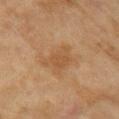– biopsy status: total-body-photography surveillance lesion; no biopsy
– TBP lesion metrics: a lesion area of about 9 mm² and a symmetry-axis asymmetry near 0.4; a mean CIELAB color near L≈48 a*≈18 b*≈34 and about 6 CIELAB-L* units darker than the surrounding skin; border irregularity of about 5 on a 0–10 scale, internal color variation of about 2.5 on a 0–10 scale, and radial color variation of about 1; an automated nevus-likeness rating near 5 out of 100 and a detector confidence of about 100 out of 100 that the crop contains a lesion
– imaging modality: ~15 mm tile from a whole-body skin photo
– illumination: cross-polarized illumination
– lesion diameter: ≈4.5 mm
– anatomic site: the right upper arm
– patient: female, aged 58 to 62